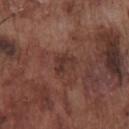The lesion was tiled from a total-body skin photograph and was not biopsied.
The subject is a male approximately 75 years of age.
A 15 mm crop from a total-body photograph taken for skin-cancer surveillance.
Automated image analysis of the tile measured a mean CIELAB color near L≈34 a*≈20 b*≈22, a lesion–skin lightness drop of about 6, and a lesion-to-skin contrast of about 6.5 (normalized; higher = more distinct). And it measured an automated nevus-likeness rating near 0 out of 100 and a detector confidence of about 100 out of 100 that the crop contains a lesion.
This is a white-light tile.
Measured at roughly 3 mm in maximum diameter.
From the chest.